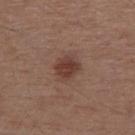<case>
  <biopsy_status>not biopsied; imaged during a skin examination</biopsy_status>
  <lighting>white-light</lighting>
  <image>
    <source>total-body photography crop</source>
    <field_of_view_mm>15</field_of_view_mm>
  </image>
  <patient>
    <sex>male</sex>
    <age_approx>55</age_approx>
  </patient>
  <automated_metrics>
    <area_mm2_approx>5.5</area_mm2_approx>
    <shape_asymmetry>0.15</shape_asymmetry>
    <cielab_L>38</cielab_L>
    <cielab_a>21</cielab_a>
    <cielab_b>24</cielab_b>
    <vs_skin_darker_L>10.0</vs_skin_darker_L>
    <vs_skin_contrast_norm>8.5</vs_skin_contrast_norm>
    <border_irregularity_0_10>1.5</border_irregularity_0_10>
    <color_variation_0_10>2.5</color_variation_0_10>
    <peripheral_color_asymmetry>1.0</peripheral_color_asymmetry>
  </automated_metrics>
  <site>right upper arm</site>
  <lesion_size>
    <long_diameter_mm_approx>2.5</long_diameter_mm_approx>
  </lesion_size>
</case>